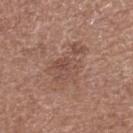Cropped from a total-body skin-imaging series; the visible field is about 15 mm. A male patient aged 73 to 77. The recorded lesion diameter is about 5 mm. The tile uses white-light illumination. Automated image analysis of the tile measured a border-irregularity rating of about 7/10 and a peripheral color-asymmetry measure near 1.5. The analysis additionally found a nevus-likeness score of about 0/100 and a detector confidence of about 100 out of 100 that the crop contains a lesion. From the leg.